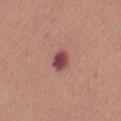Q: Where on the body is the lesion?
A: the right thigh
Q: Automated lesion metrics?
A: a lesion area of about 5 mm², a shape eccentricity near 0.55, and two-axis asymmetry of about 0.2; a border-irregularity rating of about 1.5/10 and a color-variation rating of about 4.5/10; a detector confidence of about 100 out of 100 that the crop contains a lesion
Q: How was the tile lit?
A: white-light
Q: Patient demographics?
A: female, aged around 60
Q: What is the imaging modality?
A: ~15 mm tile from a whole-body skin photo